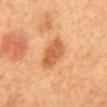Impression:
This lesion was catalogued during total-body skin photography and was not selected for biopsy.
Context:
A lesion tile, about 15 mm wide, cut from a 3D total-body photograph. On the mid back. The subject is a female aged around 60.